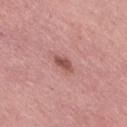Captured during whole-body skin photography for melanoma surveillance; the lesion was not biopsied.